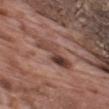notes = total-body-photography surveillance lesion; no biopsy | anatomic site = the upper back | imaging modality = total-body-photography crop, ~15 mm field of view | patient = male, aged around 70 | lighting = white-light illumination.